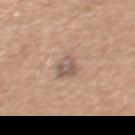Case summary:
• biopsy status — catalogued during a skin exam; not biopsied
• image source — ~15 mm tile from a whole-body skin photo
• body site — the abdomen
• automated lesion analysis — an average lesion color of about L≈56 a*≈17 b*≈24 (CIELAB), a lesion–skin lightness drop of about 9, and a normalized lesion–skin contrast near 7
• lesion size — ≈3 mm
• patient — male, roughly 55 years of age
• lighting — white-light